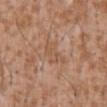imaging modality: total-body-photography crop, ~15 mm field of view
subject: male, roughly 45 years of age
anatomic site: the abdomen